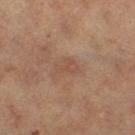Q: Automated lesion metrics?
A: an area of roughly 3.5 mm², an eccentricity of roughly 0.8, and a symmetry-axis asymmetry near 0.5; a border-irregularity rating of about 5/10, internal color variation of about 1 on a 0–10 scale, and radial color variation of about 0.5
Q: What lighting was used for the tile?
A: cross-polarized
Q: Who is the patient?
A: female, roughly 60 years of age
Q: How large is the lesion?
A: about 3 mm
Q: What kind of image is this?
A: total-body-photography crop, ~15 mm field of view
Q: What is the anatomic site?
A: the left thigh